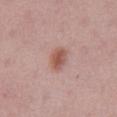This lesion was catalogued during total-body skin photography and was not selected for biopsy. A male subject in their mid- to late 60s. The lesion's longest dimension is about 3 mm. A 15 mm close-up tile from a total-body photography series done for melanoma screening. The tile uses white-light illumination. Located on the abdomen.